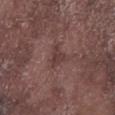Case summary:
– workup — imaged on a skin check; not biopsied
– location — the left lower leg
– subject — male, in their mid-70s
– image source — total-body-photography crop, ~15 mm field of view
– diameter — about 2.5 mm